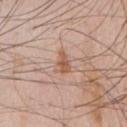The lesion-visualizer software estimated a lesion color around L≈56 a*≈21 b*≈30 in CIELAB, about 10 CIELAB-L* units darker than the surrounding skin, and a normalized lesion–skin contrast near 7.5. The analysis additionally found a border-irregularity index near 3.5/10, a within-lesion color-variation index near 2/10, and radial color variation of about 0.5. It also reported an automated nevus-likeness rating near 5 out of 100. A lesion tile, about 15 mm wide, cut from a 3D total-body photograph. On the chest. A male patient in their 60s.